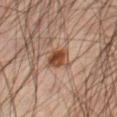Image and clinical context: Automated tile analysis of the lesion measured a shape eccentricity near 0.4 and a symmetry-axis asymmetry near 0.25. Imaged with cross-polarized lighting. The recorded lesion diameter is about 2.5 mm. This image is a 15 mm lesion crop taken from a total-body photograph. The patient is a male in their mid-40s. The lesion is on the right thigh.